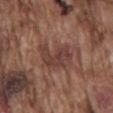biopsy_status: not biopsied; imaged during a skin examination
image:
  source: total-body photography crop
  field_of_view_mm: 15
lighting: white-light
patient:
  sex: male
  age_approx: 75
site: back
lesion_size:
  long_diameter_mm_approx: 4.0
automated_metrics:
  border_irregularity_0_10: 4.0
  color_variation_0_10: 3.0
  peripheral_color_asymmetry: 1.0